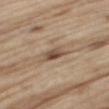The lesion is located on the right thigh.
The recorded lesion diameter is about 3 mm.
A roughly 15 mm field-of-view crop from a total-body skin photograph.
The tile uses white-light illumination.
The lesion-visualizer software estimated a mean CIELAB color near L≈50 a*≈15 b*≈28, roughly 12 lightness units darker than nearby skin, and a normalized lesion–skin contrast near 8.5. And it measured a border-irregularity index near 2/10, internal color variation of about 5.5 on a 0–10 scale, and peripheral color asymmetry of about 2.
The patient is a male aged around 70.